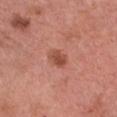Recorded during total-body skin imaging; not selected for excision or biopsy.
A lesion tile, about 15 mm wide, cut from a 3D total-body photograph.
From the front of the torso.
The subject is a female aged 58–62.